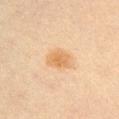Q: Was a biopsy performed?
A: imaged on a skin check; not biopsied
Q: Where on the body is the lesion?
A: the chest
Q: How was the tile lit?
A: cross-polarized illumination
Q: Patient demographics?
A: male, approximately 60 years of age
Q: What kind of image is this?
A: ~15 mm tile from a whole-body skin photo